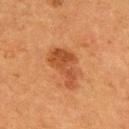Acquisition and patient details: An algorithmic analysis of the crop reported a lesion area of about 9.5 mm², a shape eccentricity near 0.85, and a symmetry-axis asymmetry near 0.55. From the upper back. A lesion tile, about 15 mm wide, cut from a 3D total-body photograph. Captured under cross-polarized illumination. A male patient aged 53–57.